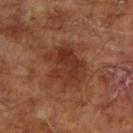Case summary:
- notes · catalogued during a skin exam; not biopsied
- patient · male, aged approximately 65
- anatomic site · the right upper arm
- acquisition · total-body-photography crop, ~15 mm field of view